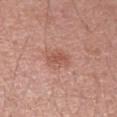Clinical impression: The lesion was tiled from a total-body skin photograph and was not biopsied. Image and clinical context: Automated tile analysis of the lesion measured a lesion area of about 5 mm² and an outline eccentricity of about 0.7 (0 = round, 1 = elongated). The analysis additionally found a mean CIELAB color near L≈55 a*≈24 b*≈29, about 8 CIELAB-L* units darker than the surrounding skin, and a normalized border contrast of about 6. The software also gave a border-irregularity index near 3/10 and a color-variation rating of about 2.5/10. The analysis additionally found an automated nevus-likeness rating near 5 out of 100 and lesion-presence confidence of about 100/100. Approximately 3 mm at its widest. A female subject, in their mid-30s. From the right upper arm. This is a white-light tile. A lesion tile, about 15 mm wide, cut from a 3D total-body photograph.